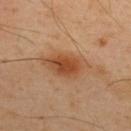{
  "biopsy_status": "not biopsied; imaged during a skin examination",
  "lesion_size": {
    "long_diameter_mm_approx": 5.5
  },
  "image": {
    "source": "total-body photography crop",
    "field_of_view_mm": 15
  },
  "site": "upper back",
  "automated_metrics": {
    "eccentricity": 0.8,
    "shape_asymmetry": 0.25,
    "cielab_L": 49,
    "cielab_a": 23,
    "cielab_b": 36,
    "vs_skin_darker_L": 10.0,
    "vs_skin_contrast_norm": 8.0,
    "border_irregularity_0_10": 2.5,
    "color_variation_0_10": 5.0,
    "peripheral_color_asymmetry": 1.5
  },
  "patient": {
    "sex": "male",
    "age_approx": 50
  },
  "lighting": "cross-polarized"
}